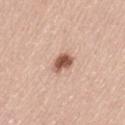biopsy status — catalogued during a skin exam; not biopsied
patient — female, aged 43–47
imaging modality — total-body-photography crop, ~15 mm field of view
location — the left thigh
lesion diameter — about 3 mm